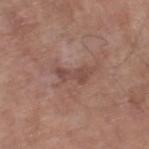biopsy status: no biopsy performed (imaged during a skin exam)
acquisition: total-body-photography crop, ~15 mm field of view
body site: the right lower leg
subject: male, aged 78–82
illumination: white-light
lesion diameter: about 4 mm